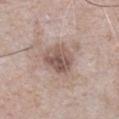Case summary:
• biopsy status · total-body-photography surveillance lesion; no biopsy
• patient · male, about 65 years old
• location · the chest
• image source · ~15 mm tile from a whole-body skin photo